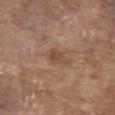Captured during whole-body skin photography for melanoma surveillance; the lesion was not biopsied. The recorded lesion diameter is about 3 mm. Automated tile analysis of the lesion measured a border-irregularity rating of about 3/10, a within-lesion color-variation index near 2/10, and peripheral color asymmetry of about 0.5. It also reported lesion-presence confidence of about 100/100. Cropped from a whole-body photographic skin survey; the tile spans about 15 mm. The subject is a male aged approximately 80. The lesion is located on the front of the torso.